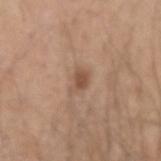Part of a total-body skin-imaging series; this lesion was reviewed on a skin check and was not flagged for biopsy.
An algorithmic analysis of the crop reported a border-irregularity rating of about 3/10, a within-lesion color-variation index near 1.5/10, and peripheral color asymmetry of about 0.5. The analysis additionally found a classifier nevus-likeness of about 70/100.
The lesion is located on the right forearm.
A male subject approximately 50 years of age.
This is a white-light tile.
A lesion tile, about 15 mm wide, cut from a 3D total-body photograph.
Approximately 3 mm at its widest.